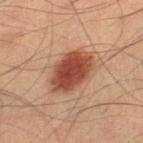The lesion was photographed on a routine skin check and not biopsied; there is no pathology result.
A close-up tile cropped from a whole-body skin photograph, about 15 mm across.
Automated image analysis of the tile measured a lesion area of about 16 mm², an eccentricity of roughly 0.75, and a symmetry-axis asymmetry near 0.15. The analysis additionally found a mean CIELAB color near L≈42 a*≈24 b*≈29, about 14 CIELAB-L* units darker than the surrounding skin, and a lesion-to-skin contrast of about 11 (normalized; higher = more distinct).
Imaged with cross-polarized lighting.
Approximately 6 mm at its widest.
Located on the right lower leg.
The patient is a male approximately 40 years of age.